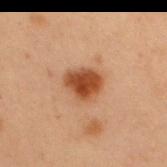  biopsy_status: not biopsied; imaged during a skin examination
  patient:
    sex: male
    age_approx: 55
  image:
    source: total-body photography crop
    field_of_view_mm: 15
  site: right upper arm
  lighting: cross-polarized